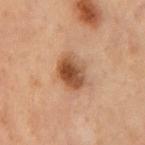follow-up = no biopsy performed (imaged during a skin exam); location = the chest; acquisition = ~15 mm crop, total-body skin-cancer survey; subject = male, about 70 years old.